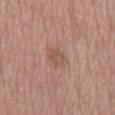follow-up — no biopsy performed (imaged during a skin exam) | subject — male, aged 78–82 | image source — ~15 mm tile from a whole-body skin photo | size — ~3 mm (longest diameter) | location — the mid back | tile lighting — white-light illumination.